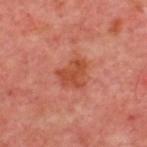| field | value |
|---|---|
| tile lighting | cross-polarized |
| patient | approximately 55 years of age |
| diameter | ~3.5 mm (longest diameter) |
| imaging modality | ~15 mm tile from a whole-body skin photo |
| body site | the back |
| automated lesion analysis | roughly 9 lightness units darker than nearby skin and a lesion-to-skin contrast of about 7.5 (normalized; higher = more distinct); border irregularity of about 3.5 on a 0–10 scale, internal color variation of about 2.5 on a 0–10 scale, and peripheral color asymmetry of about 1 |